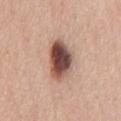follow-up: no biopsy performed (imaged during a skin exam).